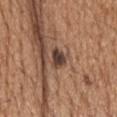follow-up = catalogued during a skin exam; not biopsied
patient = male, approximately 70 years of age
image = ~15 mm crop, total-body skin-cancer survey
size = ~2.5 mm (longest diameter)
location = the head or neck